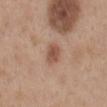Case summary:
• biopsy status · total-body-photography surveillance lesion; no biopsy
• size · ~4 mm (longest diameter)
• location · the mid back
• acquisition · ~15 mm crop, total-body skin-cancer survey
• patient · male, roughly 30 years of age
• lighting · white-light illumination